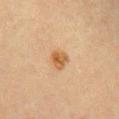  biopsy_status: not biopsied; imaged during a skin examination
  image:
    source: total-body photography crop
    field_of_view_mm: 15
  patient:
    sex: male
    age_approx: 40
  site: chest
  automated_metrics:
    area_mm2_approx: 5.0
    shape_asymmetry: 0.25
    cielab_L: 48
    cielab_a: 18
    cielab_b: 34
    vs_skin_darker_L: 9.0
    vs_skin_contrast_norm: 8.0
    border_irregularity_0_10: 2.0
    color_variation_0_10: 3.5
    peripheral_color_asymmetry: 1.0